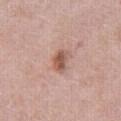The subject is a female aged around 60.
Approximately 3 mm at its widest.
A 15 mm close-up tile from a total-body photography series done for melanoma screening.
Imaged with white-light lighting.
The lesion is on the right thigh.